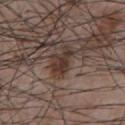biopsy status: catalogued during a skin exam; not biopsied
lighting: white-light
subject: male, aged 53 to 57
site: the chest
TBP lesion metrics: a lesion color around L≈34 a*≈16 b*≈21 in CIELAB and a normalized lesion–skin contrast near 9; a border-irregularity rating of about 4/10, a color-variation rating of about 2.5/10, and a peripheral color-asymmetry measure near 1; an automated nevus-likeness rating near 95 out of 100 and a detector confidence of about 100 out of 100 that the crop contains a lesion
lesion size: about 3.5 mm
imaging modality: ~15 mm crop, total-body skin-cancer survey